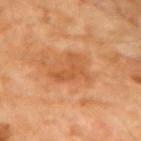Impression: Captured during whole-body skin photography for melanoma surveillance; the lesion was not biopsied. Background: On the left upper arm. The tile uses cross-polarized illumination. An algorithmic analysis of the crop reported a mean CIELAB color near L≈57 a*≈28 b*≈43, a lesion–skin lightness drop of about 8, and a normalized lesion–skin contrast near 5.5. It also reported an automated nevus-likeness rating near 5 out of 100 and a lesion-detection confidence of about 100/100. This image is a 15 mm lesion crop taken from a total-body photograph. A female subject, aged approximately 70.